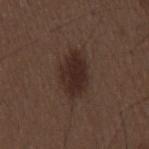Q: Is there a histopathology result?
A: imaged on a skin check; not biopsied
Q: How was this image acquired?
A: ~15 mm crop, total-body skin-cancer survey
Q: Automated lesion metrics?
A: an area of roughly 11 mm²; a lesion–skin lightness drop of about 9; border irregularity of about 2.5 on a 0–10 scale and radial color variation of about 1; a detector confidence of about 100 out of 100 that the crop contains a lesion
Q: What lighting was used for the tile?
A: white-light
Q: Who is the patient?
A: male, aged 48 to 52
Q: Lesion location?
A: the mid back
Q: What is the lesion's diameter?
A: ≈5.5 mm